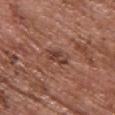Part of a total-body skin-imaging series; this lesion was reviewed on a skin check and was not flagged for biopsy.
A female subject about 65 years old.
Cropped from a total-body skin-imaging series; the visible field is about 15 mm.
The lesion is located on the upper back.
Approximately 3.5 mm at its widest.